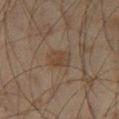biopsy_status: not biopsied; imaged during a skin examination
automated_metrics:
  area_mm2_approx: 6.5
  eccentricity: 0.65
  shape_asymmetry: 0.2
  border_irregularity_0_10: 2.0
  nevus_likeness_0_100: 20
lighting: cross-polarized
image:
  source: total-body photography crop
  field_of_view_mm: 15
lesion_size:
  long_diameter_mm_approx: 3.0
patient:
  sex: male
  age_approx: 45
site: left thigh